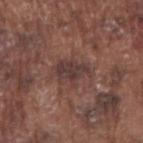A male subject aged 73–77.
Cropped from a whole-body photographic skin survey; the tile spans about 15 mm.
Automated tile analysis of the lesion measured a mean CIELAB color near L≈37 a*≈18 b*≈19, a lesion–skin lightness drop of about 8, and a normalized border contrast of about 8.
The lesion is located on the right upper arm.
About 3.5 mm across.
Captured under white-light illumination.